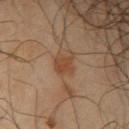notes = imaged on a skin check; not biopsied
subject = male, aged 63–67
image source = ~15 mm tile from a whole-body skin photo
illumination = cross-polarized
site = the right upper arm
automated lesion analysis = an average lesion color of about L≈42 a*≈19 b*≈30 (CIELAB), roughly 8 lightness units darker than nearby skin, and a normalized lesion–skin contrast near 7; a nevus-likeness score of about 50/100
size = about 2.5 mm